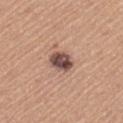Q: Was a biopsy performed?
A: total-body-photography surveillance lesion; no biopsy
Q: What is the anatomic site?
A: the left upper arm
Q: Automated lesion metrics?
A: a lesion color around L≈49 a*≈19 b*≈23 in CIELAB and a lesion-to-skin contrast of about 12 (normalized; higher = more distinct); a border-irregularity index near 1/10, internal color variation of about 5.5 on a 0–10 scale, and a peripheral color-asymmetry measure near 2
Q: Lesion size?
A: ~3 mm (longest diameter)
Q: What lighting was used for the tile?
A: white-light illumination
Q: What kind of image is this?
A: 15 mm crop, total-body photography
Q: Who is the patient?
A: female, in their mid- to late 60s